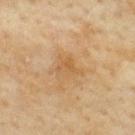Background:
Imaged with cross-polarized lighting. The lesion is located on the upper back. Measured at roughly 3.5 mm in maximum diameter. A 15 mm crop from a total-body photograph taken for skin-cancer surveillance. Automated tile analysis of the lesion measured a footprint of about 5 mm² and a shape eccentricity near 0.7. It also reported a lesion color around L≈53 a*≈17 b*≈37 in CIELAB, roughly 6 lightness units darker than nearby skin, and a normalized border contrast of about 5. It also reported border irregularity of about 4 on a 0–10 scale, internal color variation of about 3 on a 0–10 scale, and a peripheral color-asymmetry measure near 1. A female subject aged approximately 60.